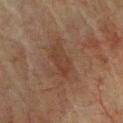Assessment: Imaged during a routine full-body skin examination; the lesion was not biopsied and no histopathology is available. Background: The patient is a male roughly 75 years of age. Cropped from a whole-body photographic skin survey; the tile spans about 15 mm. The lesion is located on the front of the torso.